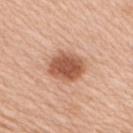This lesion was catalogued during total-body skin photography and was not selected for biopsy.
The total-body-photography lesion software estimated two-axis asymmetry of about 0.1. It also reported a mean CIELAB color near L≈56 a*≈24 b*≈33, roughly 15 lightness units darker than nearby skin, and a lesion-to-skin contrast of about 9.5 (normalized; higher = more distinct). The software also gave internal color variation of about 3.5 on a 0–10 scale and peripheral color asymmetry of about 1.
The patient is a female roughly 40 years of age.
A close-up tile cropped from a whole-body skin photograph, about 15 mm across.
Measured at roughly 4.5 mm in maximum diameter.
The lesion is located on the right upper arm.
The tile uses white-light illumination.